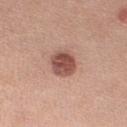Case summary:
* biopsy status — total-body-photography surveillance lesion; no biopsy
* lesion size — ≈3.5 mm
* patient — female, aged approximately 40
* acquisition — total-body-photography crop, ~15 mm field of view
* image-analysis metrics — a mean CIELAB color near L≈51 a*≈23 b*≈25, roughly 15 lightness units darker than nearby skin, and a normalized lesion–skin contrast near 10; border irregularity of about 1.5 on a 0–10 scale, a color-variation rating of about 4/10, and radial color variation of about 1.5
* site — the right upper arm
* tile lighting — white-light illumination